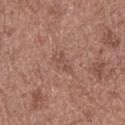| field | value |
|---|---|
| notes | no biopsy performed (imaged during a skin exam) |
| illumination | white-light |
| lesion size | ~3 mm (longest diameter) |
| imaging modality | ~15 mm crop, total-body skin-cancer survey |
| body site | the mid back |
| subject | male, aged 48–52 |
| automated metrics | a mean CIELAB color near L≈50 a*≈20 b*≈26, a lesion–skin lightness drop of about 6, and a lesion-to-skin contrast of about 4.5 (normalized; higher = more distinct) |